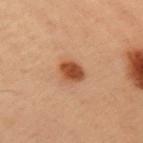No biopsy was performed on this lesion — it was imaged during a full skin examination and was not determined to be concerning. The lesion is on the upper back. This image is a 15 mm lesion crop taken from a total-body photograph. A male subject aged around 55. Automated tile analysis of the lesion measured an area of roughly 5.5 mm², a shape eccentricity near 0.45, and a shape-asymmetry score of about 0.2 (0 = symmetric). The analysis additionally found a lesion-detection confidence of about 100/100. The lesion's longest dimension is about 2.5 mm.